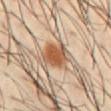biopsy_status: not biopsied; imaged during a skin examination
lighting: cross-polarized
lesion_size:
  long_diameter_mm_approx: 3.5
site: abdomen
image:
  source: total-body photography crop
  field_of_view_mm: 15
patient:
  sex: male
  age_approx: 40